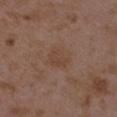Notes:
* follow-up · total-body-photography surveillance lesion; no biopsy
* patient · female, in their mid-30s
* lesion diameter · ≈3.5 mm
* imaging modality · 15 mm crop, total-body photography
* illumination · white-light
* anatomic site · the left upper arm
* automated metrics · an eccentricity of roughly 0.6 and two-axis asymmetry of about 0.25; a lesion color around L≈43 a*≈17 b*≈27 in CIELAB, a lesion–skin lightness drop of about 5, and a lesion-to-skin contrast of about 5 (normalized; higher = more distinct); radial color variation of about 1; a nevus-likeness score of about 0/100 and lesion-presence confidence of about 100/100